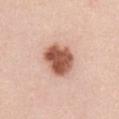Q: Was this lesion biopsied?
A: catalogued during a skin exam; not biopsied
Q: Where on the body is the lesion?
A: the chest
Q: What are the patient's age and sex?
A: female, aged approximately 40
Q: How large is the lesion?
A: ~4 mm (longest diameter)
Q: What lighting was used for the tile?
A: white-light illumination
Q: What did automated image analysis measure?
A: an area of roughly 13 mm², an eccentricity of roughly 0.55, and a symmetry-axis asymmetry near 0.2; an average lesion color of about L≈56 a*≈25 b*≈30 (CIELAB) and about 19 CIELAB-L* units darker than the surrounding skin; a border-irregularity index near 1.5/10, internal color variation of about 5 on a 0–10 scale, and radial color variation of about 2
Q: How was this image acquired?
A: total-body-photography crop, ~15 mm field of view